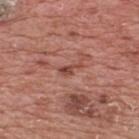Part of a total-body skin-imaging series; this lesion was reviewed on a skin check and was not flagged for biopsy.
The lesion is on the upper back.
Imaged with white-light lighting.
A 15 mm close-up extracted from a 3D total-body photography capture.
The lesion-visualizer software estimated a footprint of about 2.5 mm², a shape eccentricity near 0.9, and a symmetry-axis asymmetry near 0.6. The analysis additionally found border irregularity of about 7 on a 0–10 scale and a within-lesion color-variation index near 0/10. The software also gave lesion-presence confidence of about 95/100.
A male patient, about 70 years old.
About 3 mm across.